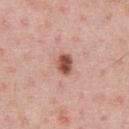Case summary:
- follow-up: no biopsy performed (imaged during a skin exam)
- tile lighting: white-light
- patient: male, in their 60s
- lesion size: about 2.5 mm
- body site: the chest
- imaging modality: ~15 mm crop, total-body skin-cancer survey